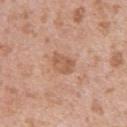Findings:
– notes — total-body-photography surveillance lesion; no biopsy
– lesion size — about 3 mm
– site — the left upper arm
– acquisition — ~15 mm tile from a whole-body skin photo
– patient — male, in their 40s
– lighting — white-light